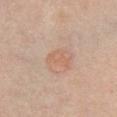This lesion was catalogued during total-body skin photography and was not selected for biopsy. Imaged with white-light lighting. An algorithmic analysis of the crop reported a border-irregularity rating of about 4/10 and a color-variation rating of about 1.5/10. Longest diameter approximately 3 mm. A lesion tile, about 15 mm wide, cut from a 3D total-body photograph. A female subject aged approximately 55. On the front of the torso.